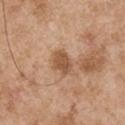Notes:
- follow-up — catalogued during a skin exam; not biopsied
- imaging modality — 15 mm crop, total-body photography
- lighting — white-light
- body site — the right upper arm
- diameter — ~3 mm (longest diameter)
- subject — male, aged 53 to 57
- automated metrics — border irregularity of about 2.5 on a 0–10 scale and a within-lesion color-variation index near 2/10